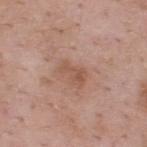No biopsy was performed on this lesion — it was imaged during a full skin examination and was not determined to be concerning. A male patient, aged 53–57. This image is a 15 mm lesion crop taken from a total-body photograph. The recorded lesion diameter is about 3.5 mm. On the upper back.